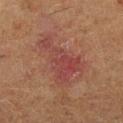| feature | finding |
|---|---|
| notes | catalogued during a skin exam; not biopsied |
| location | the left lower leg |
| subject | female, about 50 years old |
| lighting | cross-polarized |
| image | ~15 mm tile from a whole-body skin photo |
| lesion diameter | ~7.5 mm (longest diameter) |